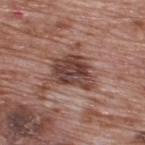A 15 mm crop from a total-body photograph taken for skin-cancer surveillance.
Longest diameter approximately 5 mm.
The lesion-visualizer software estimated an average lesion color of about L≈42 a*≈20 b*≈23 (CIELAB) and a lesion-to-skin contrast of about 10 (normalized; higher = more distinct). The software also gave border irregularity of about 4 on a 0–10 scale and internal color variation of about 5.5 on a 0–10 scale.
On the upper back.
Captured under white-light illumination.
A male patient in their 70s.